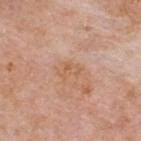| key | value |
|---|---|
| notes | total-body-photography surveillance lesion; no biopsy |
| image source | ~15 mm crop, total-body skin-cancer survey |
| patient | male, in their mid- to late 70s |
| diameter | ~2.5 mm (longest diameter) |
| lighting | white-light illumination |
| anatomic site | the upper back |